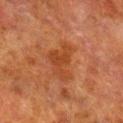Case summary:
* workup · imaged on a skin check; not biopsied
* body site · the right lower leg
* tile lighting · cross-polarized
* image · total-body-photography crop, ~15 mm field of view
* size · ~5 mm (longest diameter)
* subject · male, in their 80s